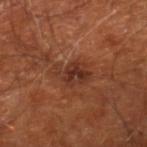The lesion was tiled from a total-body skin photograph and was not biopsied.
The patient is roughly 65 years of age.
A 15 mm close-up extracted from a 3D total-body photography capture.
The lesion's longest dimension is about 4 mm.
From the right lower leg.
Captured under cross-polarized illumination.